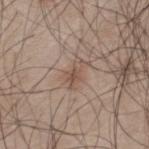Clinical impression:
No biopsy was performed on this lesion — it was imaged during a full skin examination and was not determined to be concerning.
Image and clinical context:
About 3 mm across. The lesion is on the upper back. A male patient roughly 45 years of age. A roughly 15 mm field-of-view crop from a total-body skin photograph. The tile uses white-light illumination.